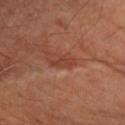| feature | finding |
|---|---|
| image | ~15 mm tile from a whole-body skin photo |
| subject | male, aged 48 to 52 |
| site | the right forearm |